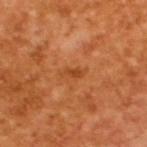A male patient roughly 65 years of age.
Automated image analysis of the tile measured a border-irregularity index near 4/10, internal color variation of about 0 on a 0–10 scale, and radial color variation of about 0. The analysis additionally found a nevus-likeness score of about 0/100 and lesion-presence confidence of about 100/100.
The lesion's longest dimension is about 3 mm.
Cropped from a total-body skin-imaging series; the visible field is about 15 mm.
The tile uses cross-polarized illumination.